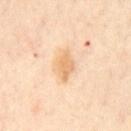Clinical impression: The lesion was tiled from a total-body skin photograph and was not biopsied. Context: A 15 mm close-up extracted from a 3D total-body photography capture. The lesion is located on the chest. The tile uses cross-polarized illumination. The subject is in their mid-50s. An algorithmic analysis of the crop reported an area of roughly 6.5 mm², an outline eccentricity of about 0.7 (0 = round, 1 = elongated), and a symmetry-axis asymmetry near 0.15.